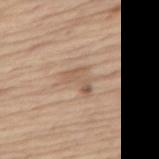Q: Is there a histopathology result?
A: catalogued during a skin exam; not biopsied
Q: Lesion size?
A: about 3.5 mm
Q: Lesion location?
A: the abdomen
Q: What did automated image analysis measure?
A: an area of roughly 5.5 mm², a shape eccentricity near 0.8, and a symmetry-axis asymmetry near 0.55; a classifier nevus-likeness of about 0/100 and lesion-presence confidence of about 100/100
Q: What lighting was used for the tile?
A: white-light
Q: What are the patient's age and sex?
A: male, aged approximately 70
Q: How was this image acquired?
A: ~15 mm tile from a whole-body skin photo